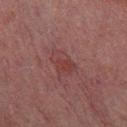Part of a total-body skin-imaging series; this lesion was reviewed on a skin check and was not flagged for biopsy.
Captured under cross-polarized illumination.
Automated tile analysis of the lesion measured a lesion color around L≈29 a*≈19 b*≈16 in CIELAB, about 4 CIELAB-L* units darker than the surrounding skin, and a normalized lesion–skin contrast near 5. The software also gave a classifier nevus-likeness of about 0/100 and a lesion-detection confidence of about 100/100.
From the leg.
The patient is a male aged around 60.
Cropped from a total-body skin-imaging series; the visible field is about 15 mm.